A male patient aged around 50, on the chest, a roughly 15 mm field-of-view crop from a total-body skin photograph.
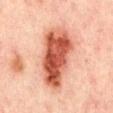Q: What is the histopathologic diagnosis?
A: a dysplastic (Clark) nevus